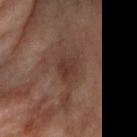workup: imaged on a skin check; not biopsied | site: the right forearm | automated lesion analysis: an area of roughly 6.5 mm², an outline eccentricity of about 0.7 (0 = round, 1 = elongated), and a symmetry-axis asymmetry near 0.3; a lesion color around L≈34 a*≈18 b*≈22 in CIELAB, a lesion–skin lightness drop of about 7, and a normalized border contrast of about 6.5; a border-irregularity rating of about 3/10, a within-lesion color-variation index near 3/10, and peripheral color asymmetry of about 1; a nevus-likeness score of about 25/100 and a lesion-detection confidence of about 100/100 | patient: female, approximately 50 years of age | image: 15 mm crop, total-body photography | illumination: cross-polarized.